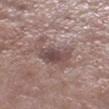  biopsy_status: not biopsied; imaged during a skin examination
  image:
    source: total-body photography crop
    field_of_view_mm: 15
  site: leg
  patient:
    sex: male
    age_approx: 55